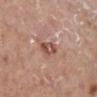An algorithmic analysis of the crop reported a mean CIELAB color near L≈51 a*≈23 b*≈26, roughly 11 lightness units darker than nearby skin, and a normalized border contrast of about 8. The software also gave a nevus-likeness score of about 0/100 and a lesion-detection confidence of about 100/100.
A 15 mm close-up extracted from a 3D total-body photography capture.
Measured at roughly 3 mm in maximum diameter.
A female patient aged approximately 75.
The lesion is on the leg.
Imaged with white-light lighting.